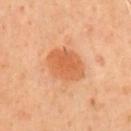Q: Was a biopsy performed?
A: imaged on a skin check; not biopsied
Q: What are the patient's age and sex?
A: male, roughly 55 years of age
Q: What kind of image is this?
A: ~15 mm crop, total-body skin-cancer survey
Q: What is the anatomic site?
A: the front of the torso